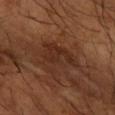Imaged during a routine full-body skin examination; the lesion was not biopsied and no histopathology is available. A male patient aged 63–67. The lesion-visualizer software estimated a lesion area of about 13 mm², a shape eccentricity near 0.65, and two-axis asymmetry of about 0.45. The tile uses cross-polarized illumination. The lesion is located on the arm. About 5 mm across. A 15 mm close-up extracted from a 3D total-body photography capture.